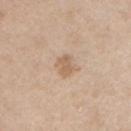{
  "biopsy_status": "not biopsied; imaged during a skin examination",
  "site": "front of the torso",
  "patient": {
    "sex": "male",
    "age_approx": 60
  },
  "lighting": "white-light",
  "lesion_size": {
    "long_diameter_mm_approx": 2.5
  },
  "automated_metrics": {
    "cielab_L": 62,
    "cielab_a": 17,
    "cielab_b": 31,
    "border_irregularity_0_10": 2.0,
    "color_variation_0_10": 2.0,
    "peripheral_color_asymmetry": 0.5,
    "lesion_detection_confidence_0_100": 100
  },
  "image": {
    "source": "total-body photography crop",
    "field_of_view_mm": 15
  }
}